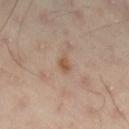Recorded during total-body skin imaging; not selected for excision or biopsy. A male patient about 55 years old. Located on the right lower leg. A lesion tile, about 15 mm wide, cut from a 3D total-body photograph.